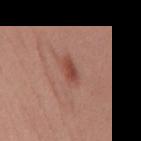follow-up — no biopsy performed (imaged during a skin exam) | site — the mid back | subject — female, roughly 25 years of age | image — ~15 mm tile from a whole-body skin photo.